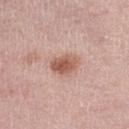Clinical impression:
This lesion was catalogued during total-body skin photography and was not selected for biopsy.
Image and clinical context:
An algorithmic analysis of the crop reported a footprint of about 7 mm², an outline eccentricity of about 0.7 (0 = round, 1 = elongated), and a shape-asymmetry score of about 0.15 (0 = symmetric). The software also gave an average lesion color of about L≈57 a*≈23 b*≈27 (CIELAB), roughly 12 lightness units darker than nearby skin, and a lesion-to-skin contrast of about 8.5 (normalized; higher = more distinct). The software also gave internal color variation of about 4.5 on a 0–10 scale and radial color variation of about 1.5. It also reported lesion-presence confidence of about 100/100. This is a white-light tile. The recorded lesion diameter is about 3.5 mm. The lesion is located on the right lower leg. A 15 mm crop from a total-body photograph taken for skin-cancer surveillance. The subject is a female roughly 70 years of age.